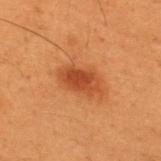The lesion is located on the upper back. The patient is a male aged 48 to 52. A lesion tile, about 15 mm wide, cut from a 3D total-body photograph.